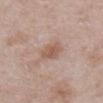- workup — no biopsy performed (imaged during a skin exam)
- lighting — white-light illumination
- location — the front of the torso
- image — ~15 mm tile from a whole-body skin photo
- lesion diameter — about 3 mm
- TBP lesion metrics — a shape eccentricity near 0.65 and a shape-asymmetry score of about 0.25 (0 = symmetric); border irregularity of about 2.5 on a 0–10 scale, internal color variation of about 2.5 on a 0–10 scale, and a peripheral color-asymmetry measure near 1; a nevus-likeness score of about 35/100 and a lesion-detection confidence of about 100/100
- patient — male, aged 53–57